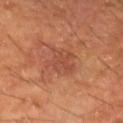acquisition = 15 mm crop, total-body photography | diameter = ≈4 mm | anatomic site = the left forearm | patient = male, roughly 60 years of age.